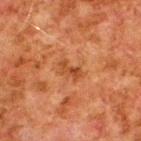body site — the upper back | automated lesion analysis — a lesion area of about 4 mm² and two-axis asymmetry of about 0.35; a mean CIELAB color near L≈38 a*≈23 b*≈33, about 7 CIELAB-L* units darker than the surrounding skin, and a normalized lesion–skin contrast near 6.5; a border-irregularity index near 4/10, a within-lesion color-variation index near 3.5/10, and radial color variation of about 1 | imaging modality — ~15 mm crop, total-body skin-cancer survey | patient — male, aged 78–82.